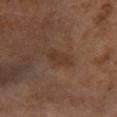Impression:
The lesion was tiled from a total-body skin photograph and was not biopsied.
Acquisition and patient details:
This image is a 15 mm lesion crop taken from a total-body photograph. Automated image analysis of the tile measured an area of roughly 4.5 mm², a shape eccentricity near 0.85, and a symmetry-axis asymmetry near 0.25. It also reported a mean CIELAB color near L≈34 a*≈18 b*≈27, about 5 CIELAB-L* units darker than the surrounding skin, and a normalized lesion–skin contrast near 5.5. It also reported a border-irregularity rating of about 3.5/10 and peripheral color asymmetry of about 0.5. It also reported a nevus-likeness score of about 0/100 and a detector confidence of about 100 out of 100 that the crop contains a lesion. On the right lower leg. The patient is a female in their mid- to late 60s. This is a cross-polarized tile.